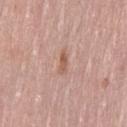workup: total-body-photography surveillance lesion; no biopsy | image: total-body-photography crop, ~15 mm field of view | subject: female, about 40 years old | size: about 2.5 mm | site: the lower back.